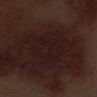  biopsy_status: not biopsied; imaged during a skin examination
  lesion_size:
    long_diameter_mm_approx: 5.5
  patient:
    sex: male
    age_approx: 70
  lighting: white-light
  automated_metrics:
    area_mm2_approx: 20.0
    eccentricity: 0.55
    nevus_likeness_0_100: 0
    lesion_detection_confidence_0_100: 90
  site: left thigh
  image:
    source: total-body photography crop
    field_of_view_mm: 15